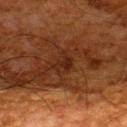No biopsy was performed on this lesion — it was imaged during a full skin examination and was not determined to be concerning.